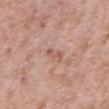Clinical impression:
The lesion was photographed on a routine skin check and not biopsied; there is no pathology result.
Image and clinical context:
Located on the mid back. Imaged with white-light lighting. Cropped from a total-body skin-imaging series; the visible field is about 15 mm. A male subject, about 55 years old. Measured at roughly 3 mm in maximum diameter.